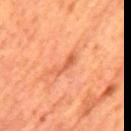Q: Is there a histopathology result?
A: total-body-photography surveillance lesion; no biopsy
Q: What lighting was used for the tile?
A: cross-polarized
Q: What is the imaging modality?
A: total-body-photography crop, ~15 mm field of view
Q: What is the lesion's diameter?
A: ≈4 mm
Q: What is the anatomic site?
A: the mid back
Q: Patient demographics?
A: male, aged approximately 65